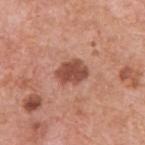Part of a total-body skin-imaging series; this lesion was reviewed on a skin check and was not flagged for biopsy. From the upper back. A male subject approximately 60 years of age. The tile uses white-light illumination. About 3.5 mm across. Cropped from a total-body skin-imaging series; the visible field is about 15 mm.